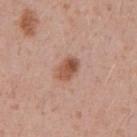Imaged during a routine full-body skin examination; the lesion was not biopsied and no histopathology is available. A male patient roughly 55 years of age. The lesion is located on the abdomen. A 15 mm close-up extracted from a 3D total-body photography capture. The lesion-visualizer software estimated a lesion area of about 6 mm², an outline eccentricity of about 0.7 (0 = round, 1 = elongated), and a symmetry-axis asymmetry near 0.2. The software also gave about 12 CIELAB-L* units darker than the surrounding skin and a normalized lesion–skin contrast near 8.5. The software also gave an automated nevus-likeness rating near 95 out of 100 and a lesion-detection confidence of about 100/100. Longest diameter approximately 3 mm.